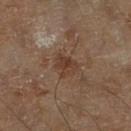The lesion was photographed on a routine skin check and not biopsied; there is no pathology result. Approximately 3 mm at its widest. A male subject, approximately 70 years of age. Cropped from a whole-body photographic skin survey; the tile spans about 15 mm. Automated tile analysis of the lesion measured border irregularity of about 3.5 on a 0–10 scale, a within-lesion color-variation index near 3/10, and radial color variation of about 1. This is a cross-polarized tile. The lesion is on the right lower leg.